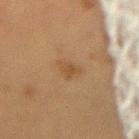Q: Is there a histopathology result?
A: imaged on a skin check; not biopsied
Q: What did automated image analysis measure?
A: border irregularity of about 3.5 on a 0–10 scale, a color-variation rating of about 1.5/10, and a peripheral color-asymmetry measure near 0.5
Q: Lesion location?
A: the back
Q: What is the imaging modality?
A: ~15 mm crop, total-body skin-cancer survey
Q: Patient demographics?
A: female, aged 48–52
Q: What lighting was used for the tile?
A: cross-polarized